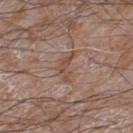Q: Lesion size?
A: about 3.5 mm
Q: Who is the patient?
A: male, approximately 60 years of age
Q: Where on the body is the lesion?
A: the right lower leg
Q: Illumination type?
A: white-light
Q: What kind of image is this?
A: total-body-photography crop, ~15 mm field of view